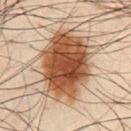Recorded during total-body skin imaging; not selected for excision or biopsy. Imaged with cross-polarized lighting. Measured at roughly 8.5 mm in maximum diameter. An algorithmic analysis of the crop reported a lesion color around L≈46 a*≈20 b*≈32 in CIELAB, roughly 18 lightness units darker than nearby skin, and a normalized border contrast of about 13. It also reported an automated nevus-likeness rating near 100 out of 100. A 15 mm close-up tile from a total-body photography series done for melanoma screening. A male subject in their 50s. From the chest.